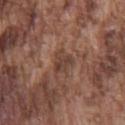follow-up = total-body-photography surveillance lesion; no biopsy
tile lighting = white-light illumination
subject = male, aged 73–77
lesion diameter = ~3 mm (longest diameter)
imaging modality = total-body-photography crop, ~15 mm field of view
anatomic site = the chest